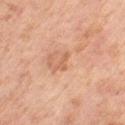The patient is a female aged 58 to 62. A 15 mm crop from a total-body photograph taken for skin-cancer surveillance. Located on the left thigh.